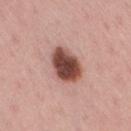{
  "biopsy_status": "not biopsied; imaged during a skin examination",
  "image": {
    "source": "total-body photography crop",
    "field_of_view_mm": 15
  },
  "automated_metrics": {
    "cielab_L": 46,
    "cielab_a": 24,
    "cielab_b": 25,
    "vs_skin_darker_L": 21.0,
    "vs_skin_contrast_norm": 13.5,
    "color_variation_0_10": 5.0,
    "peripheral_color_asymmetry": 1.5,
    "nevus_likeness_0_100": 80
  },
  "patient": {
    "sex": "female",
    "age_approx": 35
  },
  "lighting": "white-light",
  "site": "right thigh",
  "lesion_size": {
    "long_diameter_mm_approx": 4.5
  }
}